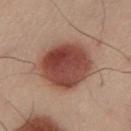{
  "biopsy_status": "not biopsied; imaged during a skin examination",
  "site": "left lower leg",
  "image": {
    "source": "total-body photography crop",
    "field_of_view_mm": 15
  },
  "lighting": "cross-polarized",
  "lesion_size": {
    "long_diameter_mm_approx": 6.5
  },
  "patient": {
    "sex": "male",
    "age_approx": 50
  }
}